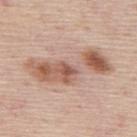biopsy_status: not biopsied; imaged during a skin examination
lighting: white-light
patient:
  sex: male
  age_approx: 45
site: mid back
lesion_size:
  long_diameter_mm_approx: 9.5
image:
  source: total-body photography crop
  field_of_view_mm: 15